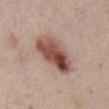image-analysis metrics: an outline eccentricity of about 0.85 (0 = round, 1 = elongated) and two-axis asymmetry of about 0.15; border irregularity of about 1.5 on a 0–10 scale, internal color variation of about 9.5 on a 0–10 scale, and peripheral color asymmetry of about 4.5; a nevus-likeness score of about 95/100 | imaging modality: 15 mm crop, total-body photography | illumination: white-light | subject: male, approximately 65 years of age | location: the chest.